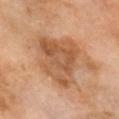{
  "site": "head or neck",
  "image": {
    "source": "total-body photography crop",
    "field_of_view_mm": 15
  },
  "patient": {
    "sex": "male",
    "age_approx": 60
  }
}